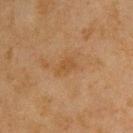Captured during whole-body skin photography for melanoma surveillance; the lesion was not biopsied. The patient is a male in their mid-40s. A 15 mm crop from a total-body photograph taken for skin-cancer surveillance. On the upper back.